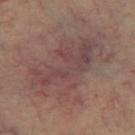Imaged during a routine full-body skin examination; the lesion was not biopsied and no histopathology is available. Cropped from a whole-body photographic skin survey; the tile spans about 15 mm. This is a cross-polarized tile. About 10.5 mm across. Located on the left thigh. The total-body-photography lesion software estimated border irregularity of about 5.5 on a 0–10 scale, a within-lesion color-variation index near 5.5/10, and peripheral color asymmetry of about 2. And it measured a detector confidence of about 95 out of 100 that the crop contains a lesion. The patient is aged around 60.